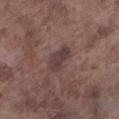<case>
<biopsy_status>not biopsied; imaged during a skin examination</biopsy_status>
<lesion_size>
  <long_diameter_mm_approx>3.5</long_diameter_mm_approx>
</lesion_size>
<lighting>white-light</lighting>
<automated_metrics>
  <area_mm2_approx>5.5</area_mm2_approx>
  <eccentricity>0.85</eccentricity>
  <shape_asymmetry>0.25</shape_asymmetry>
  <cielab_L>38</cielab_L>
  <cielab_a>16</cielab_a>
  <cielab_b>17</cielab_b>
  <vs_skin_darker_L>9.0</vs_skin_darker_L>
  <vs_skin_contrast_norm>8.0</vs_skin_contrast_norm>
  <nevus_likeness_0_100>0</nevus_likeness_0_100>
  <lesion_detection_confidence_0_100>100</lesion_detection_confidence_0_100>
</automated_metrics>
<image>
  <source>total-body photography crop</source>
  <field_of_view_mm>15</field_of_view_mm>
</image>
<patient>
  <sex>male</sex>
  <age_approx>75</age_approx>
</patient>
<site>leg</site>
</case>